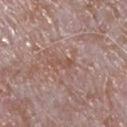biopsy status — imaged on a skin check; not biopsied
site — the chest
patient — male, aged 63–67
illumination — white-light illumination
image source — ~15 mm crop, total-body skin-cancer survey